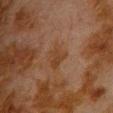Impression: This lesion was catalogued during total-body skin photography and was not selected for biopsy. Image and clinical context: A male subject aged approximately 80. An algorithmic analysis of the crop reported an area of roughly 6 mm² and a shape-asymmetry score of about 0.3 (0 = symmetric). The software also gave a lesion color around L≈31 a*≈16 b*≈26 in CIELAB, about 4 CIELAB-L* units darker than the surrounding skin, and a normalized lesion–skin contrast near 5.5. It also reported a border-irregularity index near 3/10, a within-lesion color-variation index near 2.5/10, and peripheral color asymmetry of about 1. The lesion's longest dimension is about 3 mm. Located on the chest. Captured under cross-polarized illumination. A 15 mm close-up tile from a total-body photography series done for melanoma screening.